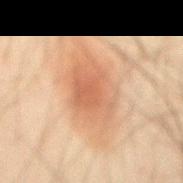image = ~15 mm crop, total-body skin-cancer survey; patient = male, aged 43 to 47; illumination = cross-polarized illumination; diameter = ~7.5 mm (longest diameter); site = the abdomen.